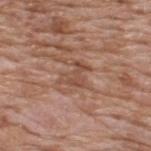Notes:
• notes: catalogued during a skin exam; not biopsied
• acquisition: ~15 mm tile from a whole-body skin photo
• image-analysis metrics: an area of roughly 6 mm² and a shape eccentricity near 0.7; a lesion color around L≈49 a*≈21 b*≈29 in CIELAB
• patient: male, roughly 60 years of age
• size: about 3.5 mm
• site: the upper back
• illumination: white-light